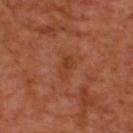<case>
  <biopsy_status>not biopsied; imaged during a skin examination</biopsy_status>
  <image>
    <source>total-body photography crop</source>
    <field_of_view_mm>15</field_of_view_mm>
  </image>
  <lesion_size>
    <long_diameter_mm_approx>3.0</long_diameter_mm_approx>
  </lesion_size>
  <patient>
    <sex>male</sex>
    <age_approx>50</age_approx>
  </patient>
  <site>upper back</site>
  <automated_metrics>
    <cielab_L>40</cielab_L>
    <cielab_a>26</cielab_a>
    <cielab_b>34</cielab_b>
    <vs_skin_contrast_norm>5.5</vs_skin_contrast_norm>
  </automated_metrics>
  <lighting>cross-polarized</lighting>
</case>